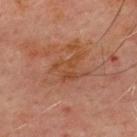Imaged during a routine full-body skin examination; the lesion was not biopsied and no histopathology is available.
Measured at roughly 3.5 mm in maximum diameter.
On the chest.
A male subject, aged 68 to 72.
Automated tile analysis of the lesion measured an area of roughly 6.5 mm² and an outline eccentricity of about 0.45 (0 = round, 1 = elongated). The software also gave a classifier nevus-likeness of about 0/100 and a lesion-detection confidence of about 100/100.
A 15 mm close-up extracted from a 3D total-body photography capture.